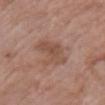Recorded during total-body skin imaging; not selected for excision or biopsy. A 15 mm crop from a total-body photograph taken for skin-cancer surveillance. Longest diameter approximately 4.5 mm. The lesion is on the left upper arm. A female patient, approximately 70 years of age. This is a white-light tile.